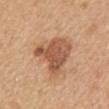Clinical impression:
Part of a total-body skin-imaging series; this lesion was reviewed on a skin check and was not flagged for biopsy.
Image and clinical context:
The subject is a female in their mid-50s. On the mid back. A 15 mm close-up extracted from a 3D total-body photography capture. Captured under white-light illumination.